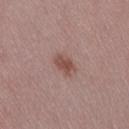Part of a total-body skin-imaging series; this lesion was reviewed on a skin check and was not flagged for biopsy.
A female subject, approximately 45 years of age.
Captured under white-light illumination.
The recorded lesion diameter is about 2.5 mm.
Located on the right thigh.
A lesion tile, about 15 mm wide, cut from a 3D total-body photograph.